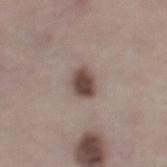Assessment: Captured during whole-body skin photography for melanoma surveillance; the lesion was not biopsied. Context: Captured under white-light illumination. The subject is a female aged 48–52. A 15 mm close-up tile from a total-body photography series done for melanoma screening. From the left lower leg.